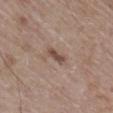<record>
  <biopsy_status>not biopsied; imaged during a skin examination</biopsy_status>
  <lighting>white-light</lighting>
  <site>right lower leg</site>
  <lesion_size>
    <long_diameter_mm_approx>2.5</long_diameter_mm_approx>
  </lesion_size>
  <patient>
    <sex>male</sex>
    <age_approx>65</age_approx>
  </patient>
  <image>
    <source>total-body photography crop</source>
    <field_of_view_mm>15</field_of_view_mm>
  </image>
</record>